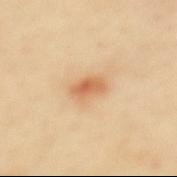No biopsy was performed on this lesion — it was imaged during a full skin examination and was not determined to be concerning. The lesion is on the mid back. A female patient aged approximately 40. A 15 mm crop from a total-body photograph taken for skin-cancer surveillance.